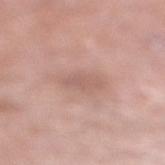– notes: catalogued during a skin exam; not biopsied
– lesion size: ~4 mm (longest diameter)
– image: ~15 mm crop, total-body skin-cancer survey
– patient: male, about 50 years old
– body site: the right lower leg
– tile lighting: white-light illumination